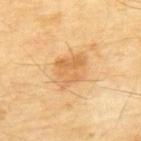Assessment:
No biopsy was performed on this lesion — it was imaged during a full skin examination and was not determined to be concerning.
Clinical summary:
A male patient, roughly 70 years of age. A close-up tile cropped from a whole-body skin photograph, about 15 mm across. The lesion is located on the upper back. Automated image analysis of the tile measured internal color variation of about 3.5 on a 0–10 scale.